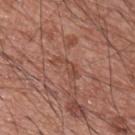  site: upper back
  lesion_size:
    long_diameter_mm_approx: 3.5
  image:
    source: total-body photography crop
    field_of_view_mm: 15
  patient:
    sex: male
    age_approx: 60